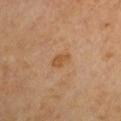Part of a total-body skin-imaging series; this lesion was reviewed on a skin check and was not flagged for biopsy. Cropped from a whole-body photographic skin survey; the tile spans about 15 mm. Located on the left arm. The patient is a male in their mid-60s. The recorded lesion diameter is about 2.5 mm. Imaged with cross-polarized lighting.